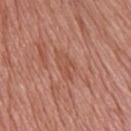Recorded during total-body skin imaging; not selected for excision or biopsy. The tile uses white-light illumination. The lesion's longest dimension is about 3.5 mm. The total-body-photography lesion software estimated a border-irregularity rating of about 4/10, a within-lesion color-variation index near 2/10, and a peripheral color-asymmetry measure near 0.5. The analysis additionally found a nevus-likeness score of about 0/100 and a lesion-detection confidence of about 80/100. A male patient aged 73 to 77. On the upper back. A close-up tile cropped from a whole-body skin photograph, about 15 mm across.